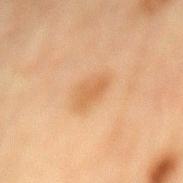Assessment: This lesion was catalogued during total-body skin photography and was not selected for biopsy. Background: Cropped from a whole-body photographic skin survey; the tile spans about 15 mm. A female patient approximately 80 years of age. Located on the mid back.